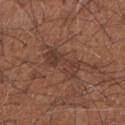The lesion was photographed on a routine skin check and not biopsied; there is no pathology result. Measured at roughly 5 mm in maximum diameter. A male subject, aged around 65. On the right forearm. A close-up tile cropped from a whole-body skin photograph, about 15 mm across. This is a white-light tile.